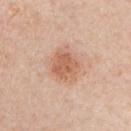Part of a total-body skin-imaging series; this lesion was reviewed on a skin check and was not flagged for biopsy. The total-body-photography lesion software estimated a footprint of about 9.5 mm² and a shape-asymmetry score of about 0.2 (0 = symmetric). The software also gave border irregularity of about 2.5 on a 0–10 scale, a within-lesion color-variation index near 3/10, and peripheral color asymmetry of about 1. The recorded lesion diameter is about 4 mm. The lesion is on the chest. A male subject, roughly 50 years of age. A 15 mm close-up extracted from a 3D total-body photography capture.